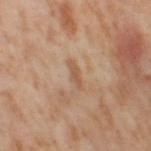Part of a total-body skin-imaging series; this lesion was reviewed on a skin check and was not flagged for biopsy.